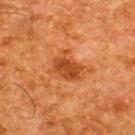<case>
<biopsy_status>not biopsied; imaged during a skin examination</biopsy_status>
<site>back</site>
<lighting>cross-polarized</lighting>
<patient>
  <sex>male</sex>
  <age_approx>65</age_approx>
</patient>
<image>
  <source>total-body photography crop</source>
  <field_of_view_mm>15</field_of_view_mm>
</image>
<lesion_size>
  <long_diameter_mm_approx>4.0</long_diameter_mm_approx>
</lesion_size>
</case>